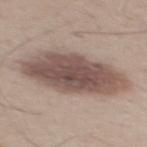Part of a total-body skin-imaging series; this lesion was reviewed on a skin check and was not flagged for biopsy. An algorithmic analysis of the crop reported a footprint of about 37 mm², an outline eccentricity of about 0.9 (0 = round, 1 = elongated), and a symmetry-axis asymmetry near 0.1. The software also gave about 14 CIELAB-L* units darker than the surrounding skin and a lesion-to-skin contrast of about 10 (normalized; higher = more distinct). Measured at roughly 10.5 mm in maximum diameter. On the mid back. A close-up tile cropped from a whole-body skin photograph, about 15 mm across. Captured under white-light illumination. A male patient, about 40 years old.